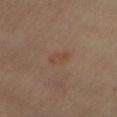Q: Was this lesion biopsied?
A: imaged on a skin check; not biopsied
Q: How was the tile lit?
A: cross-polarized illumination
Q: How large is the lesion?
A: ~3 mm (longest diameter)
Q: What did automated image analysis measure?
A: an eccentricity of roughly 0.9 and a symmetry-axis asymmetry near 0.3; border irregularity of about 3.5 on a 0–10 scale and internal color variation of about 0 on a 0–10 scale
Q: Who is the patient?
A: male, aged 68 to 72
Q: Lesion location?
A: the abdomen
Q: What kind of image is this?
A: ~15 mm tile from a whole-body skin photo